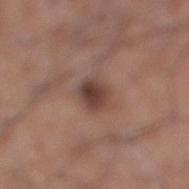biopsy status — imaged on a skin check; not biopsied | tile lighting — white-light illumination | image-analysis metrics — border irregularity of about 2 on a 0–10 scale, a color-variation rating of about 5/10, and peripheral color asymmetry of about 1.5 | site — the right lower leg | lesion diameter — ≈3 mm | image source — ~15 mm crop, total-body skin-cancer survey | subject — male, in their 60s.